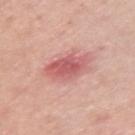Assessment: The lesion was tiled from a total-body skin photograph and was not biopsied. Clinical summary: This image is a 15 mm lesion crop taken from a total-body photograph. The lesion's longest dimension is about 4.5 mm. From the left upper arm. The tile uses white-light illumination. The patient is a female aged approximately 40.